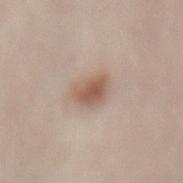Findings:
– notes · imaged on a skin check; not biopsied
– body site · the mid back
– image source · ~15 mm tile from a whole-body skin photo
– lighting · white-light illumination
– subject · female, aged around 30
– lesion size · ≈3.5 mm